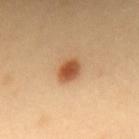{
  "biopsy_status": "not biopsied; imaged during a skin examination",
  "site": "upper back",
  "lesion_size": {
    "long_diameter_mm_approx": 3.0
  },
  "automated_metrics": {
    "eccentricity": 0.7,
    "vs_skin_darker_L": 15.0,
    "vs_skin_contrast_norm": 9.5,
    "nevus_likeness_0_100": 100
  },
  "image": {
    "source": "total-body photography crop",
    "field_of_view_mm": 15
  },
  "patient": {
    "sex": "female",
    "age_approx": 40
  }
}